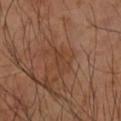| key | value |
|---|---|
| follow-up | no biopsy performed (imaged during a skin exam) |
| imaging modality | ~15 mm crop, total-body skin-cancer survey |
| tile lighting | cross-polarized |
| site | the right forearm |